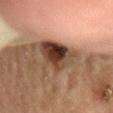Q: Was a biopsy performed?
A: total-body-photography surveillance lesion; no biopsy
Q: What is the imaging modality?
A: ~15 mm tile from a whole-body skin photo
Q: Lesion size?
A: about 6 mm
Q: Lesion location?
A: the mid back
Q: Patient demographics?
A: male, about 85 years old or older
Q: Automated lesion metrics?
A: a footprint of about 15 mm², an outline eccentricity of about 0.7 (0 = round, 1 = elongated), and two-axis asymmetry of about 0.35; a mean CIELAB color near L≈34 a*≈16 b*≈25 and about 16 CIELAB-L* units darker than the surrounding skin; a within-lesion color-variation index near 9.5/10 and a peripheral color-asymmetry measure near 3.5; a classifier nevus-likeness of about 95/100 and a detector confidence of about 70 out of 100 that the crop contains a lesion
Q: How was the tile lit?
A: cross-polarized illumination